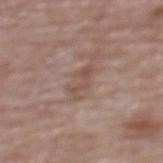* biopsy status: imaged on a skin check; not biopsied
* lesion diameter: ~3.5 mm (longest diameter)
* illumination: white-light illumination
* subject: female, in their mid-60s
* body site: the upper back
* image: total-body-photography crop, ~15 mm field of view